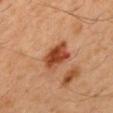workup = imaged on a skin check; not biopsied | tile lighting = cross-polarized | acquisition = ~15 mm crop, total-body skin-cancer survey | diameter = about 3.5 mm | site = the mid back | subject = male, in their mid- to late 40s.